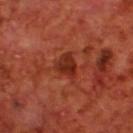Located on the upper back. A male subject, aged 68–72. The lesion-visualizer software estimated a lesion color around L≈30 a*≈30 b*≈32 in CIELAB, a lesion–skin lightness drop of about 9, and a normalized border contrast of about 8.5. A roughly 15 mm field-of-view crop from a total-body skin photograph.